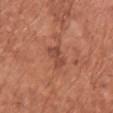Case summary:
– illumination — white-light
– image — ~15 mm crop, total-body skin-cancer survey
– site — the upper back
– patient — female, aged around 75